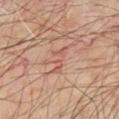<case>
  <biopsy_status>not biopsied; imaged during a skin examination</biopsy_status>
  <site>chest</site>
  <image>
    <source>total-body photography crop</source>
    <field_of_view_mm>15</field_of_view_mm>
  </image>
  <patient>
    <age_approx>65</age_approx>
  </patient>
</case>